Automated image analysis of the tile measured border irregularity of about 6.5 on a 0–10 scale and radial color variation of about 3; located on the abdomen; a male subject, in their mid- to late 60s; imaged with white-light lighting; a 15 mm close-up extracted from a 3D total-body photography capture: 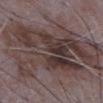Biopsy histopathology demonstrated a solar lentigo — a benign lesion.